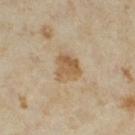The lesion was photographed on a routine skin check and not biopsied; there is no pathology result. Imaged with cross-polarized lighting. The subject is a female about 35 years old. A roughly 15 mm field-of-view crop from a total-body skin photograph. The lesion is on the leg.